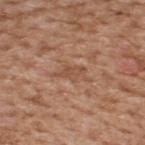  patient:
    sex: male
    age_approx: 65
  site: upper back
  image:
    source: total-body photography crop
    field_of_view_mm: 15
  lesion_size:
    long_diameter_mm_approx: 3.0